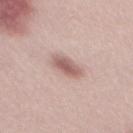<lesion>
<biopsy_status>not biopsied; imaged during a skin examination</biopsy_status>
<image>
  <source>total-body photography crop</source>
  <field_of_view_mm>15</field_of_view_mm>
</image>
<lighting>white-light</lighting>
<patient>
  <sex>male</sex>
  <age_approx>30</age_approx>
</patient>
<lesion_size>
  <long_diameter_mm_approx>4.0</long_diameter_mm_approx>
</lesion_size>
</lesion>